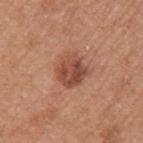Imaged during a routine full-body skin examination; the lesion was not biopsied and no histopathology is available.
The subject is a female approximately 50 years of age.
Approximately 4 mm at its widest.
The lesion-visualizer software estimated a footprint of about 9.5 mm² and a shape-asymmetry score of about 0.15 (0 = symmetric). The software also gave a mean CIELAB color near L≈48 a*≈25 b*≈30 and about 12 CIELAB-L* units darker than the surrounding skin.
A lesion tile, about 15 mm wide, cut from a 3D total-body photograph.
The lesion is on the left upper arm.
Imaged with white-light lighting.